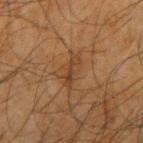| key | value |
|---|---|
| follow-up | total-body-photography surveillance lesion; no biopsy |
| patient | male, aged around 65 |
| size | ~3 mm (longest diameter) |
| imaging modality | 15 mm crop, total-body photography |
| location | the right upper arm |